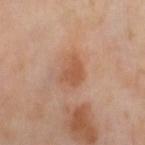Q: Is there a histopathology result?
A: imaged on a skin check; not biopsied
Q: What are the patient's age and sex?
A: female, about 50 years old
Q: What kind of image is this?
A: 15 mm crop, total-body photography
Q: What is the anatomic site?
A: the right thigh
Q: Automated lesion metrics?
A: a nevus-likeness score of about 30/100
Q: What is the lesion's diameter?
A: ~3.5 mm (longest diameter)
Q: Illumination type?
A: cross-polarized illumination